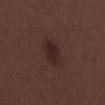Impression:
Recorded during total-body skin imaging; not selected for excision or biopsy.
Context:
Located on the mid back. The patient is a male aged around 30. A lesion tile, about 15 mm wide, cut from a 3D total-body photograph.